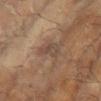automated metrics: a mean CIELAB color near L≈41 a*≈14 b*≈23, about 6 CIELAB-L* units darker than the surrounding skin, and a normalized lesion–skin contrast near 5.5; a nevus-likeness score of about 0/100 and a detector confidence of about 85 out of 100 that the crop contains a lesion
diameter: ≈4 mm
acquisition: ~15 mm tile from a whole-body skin photo
patient: female, aged approximately 80
illumination: cross-polarized
site: the left arm